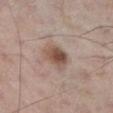Clinical impression: The lesion was photographed on a routine skin check and not biopsied; there is no pathology result. Background: Automated tile analysis of the lesion measured a lesion area of about 7 mm² and a shape eccentricity near 0.4. The software also gave lesion-presence confidence of about 100/100. The lesion is located on the left lower leg. Cropped from a total-body skin-imaging series; the visible field is about 15 mm. The patient is a male roughly 60 years of age. Longest diameter approximately 3 mm. Captured under white-light illumination.